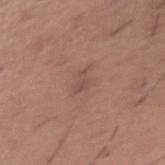<record>
  <biopsy_status>not biopsied; imaged during a skin examination</biopsy_status>
  <lesion_size>
    <long_diameter_mm_approx>3.0</long_diameter_mm_approx>
  </lesion_size>
  <image>
    <source>total-body photography crop</source>
    <field_of_view_mm>15</field_of_view_mm>
  </image>
  <lighting>white-light</lighting>
  <site>abdomen</site>
  <patient>
    <sex>male</sex>
    <age_approx>45</age_approx>
  </patient>
</record>